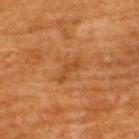Notes:
* workup · catalogued during a skin exam; not biopsied
* size · ≈3.5 mm
* lighting · cross-polarized
* patient · male, about 60 years old
* anatomic site · the upper back
* image · ~15 mm crop, total-body skin-cancer survey